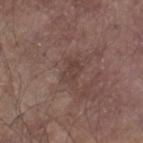{"biopsy_status": "not biopsied; imaged during a skin examination", "site": "right lower leg", "patient": {"sex": "male", "age_approx": 80}, "automated_metrics": {"cielab_L": 39, "cielab_a": 17, "cielab_b": 20, "vs_skin_darker_L": 6.0, "border_irregularity_0_10": 2.5, "color_variation_0_10": 2.0, "peripheral_color_asymmetry": 0.5, "nevus_likeness_0_100": 0}, "lesion_size": {"long_diameter_mm_approx": 2.5}, "image": {"source": "total-body photography crop", "field_of_view_mm": 15}}